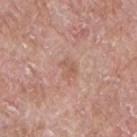| field | value |
|---|---|
| notes | total-body-photography surveillance lesion; no biopsy |
| acquisition | 15 mm crop, total-body photography |
| patient | male, approximately 65 years of age |
| diameter | ~2.5 mm (longest diameter) |
| site | the front of the torso |
| automated metrics | an outline eccentricity of about 0.7 (0 = round, 1 = elongated); a lesion–skin lightness drop of about 7 and a lesion-to-skin contrast of about 5 (normalized; higher = more distinct); a nevus-likeness score of about 0/100 and a lesion-detection confidence of about 100/100 |